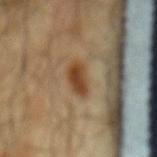{
  "biopsy_status": "not biopsied; imaged during a skin examination"
}